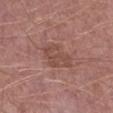Captured during whole-body skin photography for melanoma surveillance; the lesion was not biopsied.
About 4 mm across.
A 15 mm crop from a total-body photograph taken for skin-cancer surveillance.
An algorithmic analysis of the crop reported lesion-presence confidence of about 100/100.
This is a white-light tile.
From the right lower leg.
A male patient aged approximately 55.